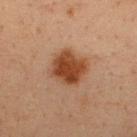{"biopsy_status": "not biopsied; imaged during a skin examination", "lighting": "cross-polarized", "lesion_size": {"long_diameter_mm_approx": 4.0}, "automated_metrics": {"border_irregularity_0_10": 1.5, "color_variation_0_10": 4.0, "peripheral_color_asymmetry": 1.0, "nevus_likeness_0_100": 100, "lesion_detection_confidence_0_100": 100}, "site": "upper back", "patient": {"sex": "male", "age_approx": 35}, "image": {"source": "total-body photography crop", "field_of_view_mm": 15}}